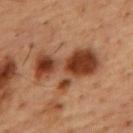Measured at roughly 7.5 mm in maximum diameter.
A 15 mm crop from a total-body photograph taken for skin-cancer surveillance.
A male patient aged 53–57.
On the upper back.
The tile uses cross-polarized illumination.
An algorithmic analysis of the crop reported a footprint of about 24 mm² and two-axis asymmetry of about 0.45. It also reported internal color variation of about 8.5 on a 0–10 scale and radial color variation of about 3.5.